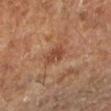Imaged during a routine full-body skin examination; the lesion was not biopsied and no histopathology is available.
Located on the left lower leg.
Captured under cross-polarized illumination.
A 15 mm crop from a total-body photograph taken for skin-cancer surveillance.
A patient in their mid-60s.